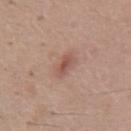| field | value |
|---|---|
| notes | catalogued during a skin exam; not biopsied |
| patient | female, approximately 65 years of age |
| anatomic site | the chest |
| imaging modality | total-body-photography crop, ~15 mm field of view |
| lesion size | ≈3 mm |